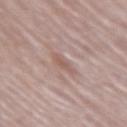  biopsy_status: not biopsied; imaged during a skin examination
  image:
    source: total-body photography crop
    field_of_view_mm: 15
  patient:
    sex: female
    age_approx: 65
  site: right upper arm